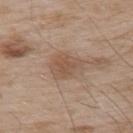notes — imaged on a skin check; not biopsied
tile lighting — white-light
anatomic site — the upper back
subject — male, aged around 55
lesion diameter — ≈4 mm
image source — 15 mm crop, total-body photography